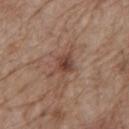Clinical impression: Recorded during total-body skin imaging; not selected for excision or biopsy. Background: A male patient in their 70s. Longest diameter approximately 2.5 mm. This image is a 15 mm lesion crop taken from a total-body photograph. The lesion is located on the mid back. This is a white-light tile.